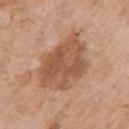Clinical impression:
The lesion was tiled from a total-body skin photograph and was not biopsied.
Context:
This is a white-light tile. The lesion is on the right upper arm. A 15 mm crop from a total-body photograph taken for skin-cancer surveillance. A female patient, in their mid-70s.